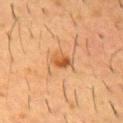<tbp_lesion>
  <biopsy_status>not biopsied; imaged during a skin examination</biopsy_status>
  <site>chest</site>
  <patient>
    <sex>male</sex>
    <age_approx>55</age_approx>
  </patient>
  <lighting>cross-polarized</lighting>
  <automated_metrics>
    <cielab_L>49</cielab_L>
    <cielab_a>23</cielab_a>
    <cielab_b>38</cielab_b>
    <vs_skin_darker_L>10.0</vs_skin_darker_L>
    <vs_skin_contrast_norm>7.5</vs_skin_contrast_norm>
    <border_irregularity_0_10>2.0</border_irregularity_0_10>
    <color_variation_0_10>5.5</color_variation_0_10>
    <peripheral_color_asymmetry>2.0</peripheral_color_asymmetry>
  </automated_metrics>
  <image>
    <source>total-body photography crop</source>
    <field_of_view_mm>15</field_of_view_mm>
  </image>
  <lesion_size>
    <long_diameter_mm_approx>2.5</long_diameter_mm_approx>
  </lesion_size>
</tbp_lesion>